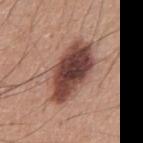A male patient, aged 58–62. Located on the abdomen. This is a white-light tile. This image is a 15 mm lesion crop taken from a total-body photograph. The lesion-visualizer software estimated an automated nevus-likeness rating near 20 out of 100 and a detector confidence of about 100 out of 100 that the crop contains a lesion.